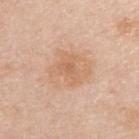* notes: no biopsy performed (imaged during a skin exam)
* patient: female, aged approximately 45
* anatomic site: the right upper arm
* image source: ~15 mm crop, total-body skin-cancer survey
* diameter: ~5 mm (longest diameter)
* lighting: white-light
* automated lesion analysis: a lesion area of about 17 mm² and two-axis asymmetry of about 0.2; an average lesion color of about L≈66 a*≈19 b*≈33 (CIELAB) and a lesion-to-skin contrast of about 5.5 (normalized; higher = more distinct); a border-irregularity index near 2.5/10, a color-variation rating of about 3.5/10, and peripheral color asymmetry of about 1